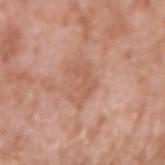Assessment:
The lesion was tiled from a total-body skin photograph and was not biopsied.
Context:
Automated tile analysis of the lesion measured a classifier nevus-likeness of about 0/100 and a detector confidence of about 100 out of 100 that the crop contains a lesion. A male subject aged around 60. Cropped from a total-body skin-imaging series; the visible field is about 15 mm. The recorded lesion diameter is about 4.5 mm. Located on the right upper arm. The tile uses white-light illumination.